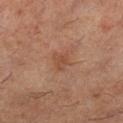The lesion was tiled from a total-body skin photograph and was not biopsied.
The lesion is located on the left lower leg.
This image is a 15 mm lesion crop taken from a total-body photograph.
The tile uses cross-polarized illumination.
Automated tile analysis of the lesion measured a lesion area of about 4.5 mm² and a shape eccentricity near 0.65. It also reported a border-irregularity rating of about 3/10, internal color variation of about 1 on a 0–10 scale, and radial color variation of about 0.5.
The recorded lesion diameter is about 2.5 mm.
The subject is a male approximately 60 years of age.